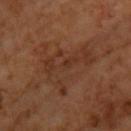Assessment: Recorded during total-body skin imaging; not selected for excision or biopsy. Image and clinical context: Located on the upper back. The patient is a male aged around 65. Captured under cross-polarized illumination. The recorded lesion diameter is about 7.5 mm. Cropped from a total-body skin-imaging series; the visible field is about 15 mm.